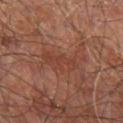The lesion was photographed on a routine skin check and not biopsied; there is no pathology result. A male subject, aged approximately 60. Cropped from a total-body skin-imaging series; the visible field is about 15 mm. The recorded lesion diameter is about 5 mm. Located on the right leg.